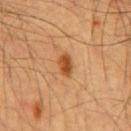{"biopsy_status": "not biopsied; imaged during a skin examination", "image": {"source": "total-body photography crop", "field_of_view_mm": 15}, "lighting": "cross-polarized", "patient": {"sex": "male", "age_approx": 60}, "site": "front of the torso", "lesion_size": {"long_diameter_mm_approx": 2.5}}